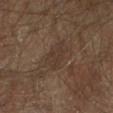image:
  source: total-body photography crop
  field_of_view_mm: 15
lighting: cross-polarized
site: arm
lesion_size:
  long_diameter_mm_approx: 4.0
patient:
  sex: male
  age_approx: 65